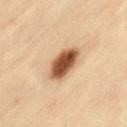notes: no biopsy performed (imaged during a skin exam)
diameter: ~5 mm (longest diameter)
site: the lower back
automated metrics: an outline eccentricity of about 0.85 (0 = round, 1 = elongated) and two-axis asymmetry of about 0.2; border irregularity of about 2 on a 0–10 scale, a within-lesion color-variation index near 5.5/10, and a peripheral color-asymmetry measure near 1.5; a classifier nevus-likeness of about 100/100 and lesion-presence confidence of about 100/100
tile lighting: cross-polarized illumination
imaging modality: ~15 mm crop, total-body skin-cancer survey
subject: female, aged 43–47